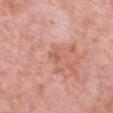notes=imaged on a skin check; not biopsied
automated lesion analysis=a normalized border contrast of about 5; a classifier nevus-likeness of about 0/100 and lesion-presence confidence of about 100/100
acquisition=total-body-photography crop, ~15 mm field of view
lighting=white-light illumination
diameter=~1.5 mm (longest diameter)
subject=female, aged 48–52
location=the chest